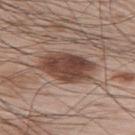Context:
The lesion's longest dimension is about 6.5 mm. The tile uses white-light illumination. The total-body-photography lesion software estimated a lesion color around L≈44 a*≈19 b*≈25 in CIELAB, about 15 CIELAB-L* units darker than the surrounding skin, and a normalized lesion–skin contrast near 11. A male patient roughly 65 years of age. The lesion is on the upper back. Cropped from a whole-body photographic skin survey; the tile spans about 15 mm.